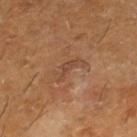{"image": {"source": "total-body photography crop", "field_of_view_mm": 15}, "lighting": "cross-polarized", "automated_metrics": {"area_mm2_approx": 4.5, "eccentricity": 0.95, "shape_asymmetry": 0.5, "cielab_L": 42, "cielab_a": 19, "cielab_b": 29, "vs_skin_darker_L": 6.0, "vs_skin_contrast_norm": 5.5, "border_irregularity_0_10": 7.0, "color_variation_0_10": 0.0, "peripheral_color_asymmetry": 0.0, "lesion_detection_confidence_0_100": 100}, "site": "right lower leg", "patient": {"sex": "male", "age_approx": 60}}